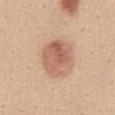Assessment:
No biopsy was performed on this lesion — it was imaged during a full skin examination and was not determined to be concerning.
Context:
From the abdomen. Measured at roughly 5 mm in maximum diameter. A female subject roughly 40 years of age. Automated tile analysis of the lesion measured a footprint of about 16 mm², an outline eccentricity of about 0.65 (0 = round, 1 = elongated), and a shape-asymmetry score of about 0.15 (0 = symmetric). And it measured an average lesion color of about L≈61 a*≈22 b*≈31 (CIELAB) and a lesion-to-skin contrast of about 7 (normalized; higher = more distinct). And it measured a border-irregularity rating of about 1.5/10 and a color-variation rating of about 5.5/10. And it measured a nevus-likeness score of about 95/100. Imaged with white-light lighting. A lesion tile, about 15 mm wide, cut from a 3D total-body photograph.